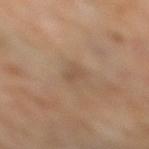| key | value |
|---|---|
| notes | imaged on a skin check; not biopsied |